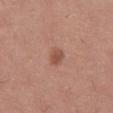The total-body-photography lesion software estimated a symmetry-axis asymmetry near 0.2. The analysis additionally found a border-irregularity rating of about 2/10, a color-variation rating of about 2/10, and radial color variation of about 0.5. The analysis additionally found a classifier nevus-likeness of about 65/100.
The lesion is located on the right thigh.
A 15 mm close-up extracted from a 3D total-body photography capture.
The subject is a female aged around 40.
Measured at roughly 3 mm in maximum diameter.
Imaged with white-light lighting.